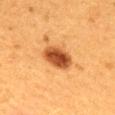notes = catalogued during a skin exam; not biopsied | lesion size = ≈4.5 mm | automated lesion analysis = a mean CIELAB color near L≈43 a*≈25 b*≈38 and about 16 CIELAB-L* units darker than the surrounding skin | patient = female, aged approximately 40 | imaging modality = total-body-photography crop, ~15 mm field of view | location = the mid back.